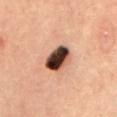workup: no biopsy performed (imaged during a skin exam)
patient: male, aged around 70
anatomic site: the mid back
tile lighting: cross-polarized illumination
acquisition: total-body-photography crop, ~15 mm field of view
diameter: about 4 mm
TBP lesion metrics: a shape eccentricity near 0.75 and a shape-asymmetry score of about 0.2 (0 = symmetric); border irregularity of about 2 on a 0–10 scale and radial color variation of about 4.5